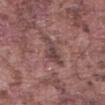workup: no biopsy performed (imaged during a skin exam)
size: ~4.5 mm (longest diameter)
patient: male, aged 73 to 77
lighting: white-light
automated lesion analysis: an area of roughly 5.5 mm² and a shape eccentricity near 0.9; a lesion–skin lightness drop of about 9 and a lesion-to-skin contrast of about 7.5 (normalized; higher = more distinct); a border-irregularity rating of about 6/10, a within-lesion color-variation index near 2.5/10, and a peripheral color-asymmetry measure near 0.5
image: 15 mm crop, total-body photography
anatomic site: the abdomen